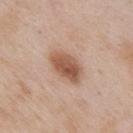Imaged during a routine full-body skin examination; the lesion was not biopsied and no histopathology is available. Longest diameter approximately 4.5 mm. Located on the chest. A male patient in their mid- to late 50s. Imaged with white-light lighting. Cropped from a whole-body photographic skin survey; the tile spans about 15 mm.